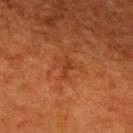Captured during whole-body skin photography for melanoma surveillance; the lesion was not biopsied.
The lesion-visualizer software estimated a shape eccentricity near 0.9 and a symmetry-axis asymmetry near 0.4. It also reported a border-irregularity rating of about 4.5/10, a within-lesion color-variation index near 0/10, and a peripheral color-asymmetry measure near 0. And it measured a nevus-likeness score of about 0/100.
A male subject approximately 60 years of age.
A 15 mm close-up extracted from a 3D total-body photography capture.
The lesion is located on the upper back.
Imaged with cross-polarized lighting.